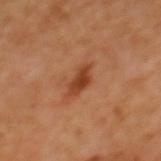| feature | finding |
|---|---|
| biopsy status | catalogued during a skin exam; not biopsied |